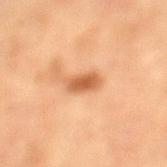Q: Is there a histopathology result?
A: no biopsy performed (imaged during a skin exam)
Q: How large is the lesion?
A: about 4 mm
Q: Who is the patient?
A: female, in their mid- to late 50s
Q: What is the imaging modality?
A: total-body-photography crop, ~15 mm field of view
Q: What is the anatomic site?
A: the leg
Q: What lighting was used for the tile?
A: cross-polarized illumination